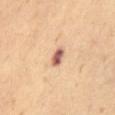| feature | finding |
|---|---|
| biopsy status | catalogued during a skin exam; not biopsied |
| subject | male, aged 63 to 67 |
| lighting | cross-polarized |
| TBP lesion metrics | a footprint of about 4 mm², an eccentricity of roughly 0.8, and a shape-asymmetry score of about 0.25 (0 = symmetric); a border-irregularity rating of about 2/10 and peripheral color asymmetry of about 1 |
| diameter | about 2.5 mm |
| image source | ~15 mm tile from a whole-body skin photo |